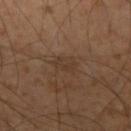  patient:
    sex: male
    age_approx: 55
  image:
    source: total-body photography crop
    field_of_view_mm: 15
  lighting: cross-polarized
  site: arm
  lesion_size:
    long_diameter_mm_approx: 2.5